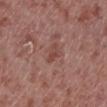Q: Where on the body is the lesion?
A: the left lower leg
Q: How large is the lesion?
A: ~3 mm (longest diameter)
Q: Automated lesion metrics?
A: a shape eccentricity near 0.9 and two-axis asymmetry of about 0.35; a border-irregularity index near 4.5/10
Q: What are the patient's age and sex?
A: male, roughly 55 years of age
Q: How was this image acquired?
A: total-body-photography crop, ~15 mm field of view